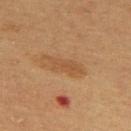The subject is a male in their 60s. On the mid back. A 15 mm close-up extracted from a 3D total-body photography capture.